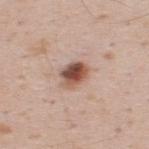  biopsy_status: not biopsied; imaged during a skin examination
  lesion_size:
    long_diameter_mm_approx: 3.0
  lighting: white-light
  automated_metrics:
    area_mm2_approx: 6.5
    eccentricity: 0.65
    shape_asymmetry: 0.2
    cielab_L: 51
    cielab_a: 21
    cielab_b: 27
    vs_skin_darker_L: 17.0
    vs_skin_contrast_norm: 11.5
    lesion_detection_confidence_0_100: 100
  image:
    source: total-body photography crop
    field_of_view_mm: 15
  patient:
    sex: male
    age_approx: 35
  site: back